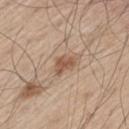Image and clinical context:
A 15 mm close-up tile from a total-body photography series done for melanoma screening. A male patient approximately 60 years of age. Longest diameter approximately 3 mm. The lesion is located on the leg. The total-body-photography lesion software estimated an eccentricity of roughly 0.75. The analysis additionally found a nevus-likeness score of about 75/100 and lesion-presence confidence of about 100/100. This is a white-light tile.